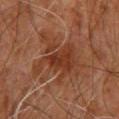{
  "biopsy_status": "not biopsied; imaged during a skin examination",
  "image": {
    "source": "total-body photography crop",
    "field_of_view_mm": 15
  },
  "lighting": "cross-polarized",
  "automated_metrics": {
    "cielab_L": 34,
    "cielab_a": 22,
    "cielab_b": 29,
    "vs_skin_darker_L": 7.0,
    "vs_skin_contrast_norm": 6.5,
    "border_irregularity_0_10": 9.0,
    "color_variation_0_10": 4.0,
    "peripheral_color_asymmetry": 1.5,
    "nevus_likeness_0_100": 0
  },
  "lesion_size": {
    "long_diameter_mm_approx": 6.5
  },
  "site": "upper back",
  "patient": {
    "sex": "male",
    "age_approx": 60
  }
}